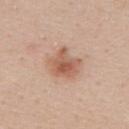notes: no biopsy performed (imaged during a skin exam) | image: ~15 mm tile from a whole-body skin photo | tile lighting: white-light illumination | anatomic site: the upper back | subject: female, aged around 45.